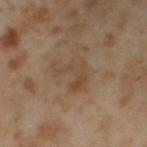The lesion was photographed on a routine skin check and not biopsied; there is no pathology result.
An algorithmic analysis of the crop reported a lesion area of about 9 mm² and two-axis asymmetry of about 0.6. The analysis additionally found a mean CIELAB color near L≈46 a*≈15 b*≈30, a lesion–skin lightness drop of about 6, and a normalized lesion–skin contrast near 5.5. And it measured a border-irregularity index near 7.5/10.
Longest diameter approximately 4 mm.
Cropped from a whole-body photographic skin survey; the tile spans about 15 mm.
On the left thigh.
The subject is a female aged 53 to 57.